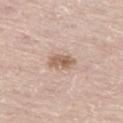The lesion was tiled from a total-body skin photograph and was not biopsied.
A female subject about 45 years old.
This image is a 15 mm lesion crop taken from a total-body photograph.
This is a white-light tile.
Automated image analysis of the tile measured an area of roughly 4.5 mm², an outline eccentricity of about 0.8 (0 = round, 1 = elongated), and a symmetry-axis asymmetry near 0.25. The software also gave border irregularity of about 3 on a 0–10 scale, a color-variation rating of about 3/10, and a peripheral color-asymmetry measure near 1.
Longest diameter approximately 3 mm.
The lesion is on the left thigh.